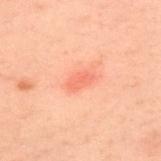Notes:
- notes: total-body-photography surveillance lesion; no biopsy
- automated lesion analysis: a lesion area of about 4 mm² and an outline eccentricity of about 0.85 (0 = round, 1 = elongated); a lesion color around L≈55 a*≈28 b*≈31 in CIELAB, a lesion–skin lightness drop of about 7, and a normalized border contrast of about 5; a nevus-likeness score of about 85/100 and a lesion-detection confidence of about 100/100
- body site: the upper back
- patient: male, aged 38 to 42
- size: ~3 mm (longest diameter)
- acquisition: ~15 mm tile from a whole-body skin photo
- illumination: cross-polarized illumination